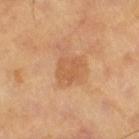notes = catalogued during a skin exam; not biopsied
illumination = cross-polarized illumination
imaging modality = total-body-photography crop, ~15 mm field of view
patient = male, roughly 65 years of age
anatomic site = the left leg